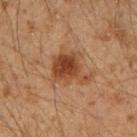Recorded during total-body skin imaging; not selected for excision or biopsy. This is a cross-polarized tile. A male patient, about 50 years old. Cropped from a whole-body photographic skin survey; the tile spans about 15 mm. On the right upper arm. Approximately 4.5 mm at its widest.